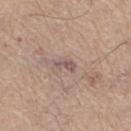{
  "biopsy_status": "not biopsied; imaged during a skin examination",
  "lesion_size": {
    "long_diameter_mm_approx": 3.0
  },
  "patient": {
    "sex": "male",
    "age_approx": 65
  },
  "lighting": "white-light",
  "site": "right thigh",
  "image": {
    "source": "total-body photography crop",
    "field_of_view_mm": 15
  }
}